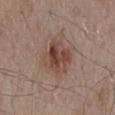Assessment:
No biopsy was performed on this lesion — it was imaged during a full skin examination and was not determined to be concerning.
Image and clinical context:
The lesion is on the back. A male patient aged around 55. A roughly 15 mm field-of-view crop from a total-body skin photograph. Measured at roughly 4 mm in maximum diameter.